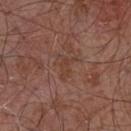The lesion was tiled from a total-body skin photograph and was not biopsied. The lesion is located on the chest. A male subject in their mid-50s. Cropped from a total-body skin-imaging series; the visible field is about 15 mm. Longest diameter approximately 3 mm. Imaged with white-light lighting.